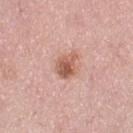Q: Is there a histopathology result?
A: imaged on a skin check; not biopsied
Q: Where on the body is the lesion?
A: the left thigh
Q: Patient demographics?
A: female, in their 50s
Q: How was this image acquired?
A: total-body-photography crop, ~15 mm field of view